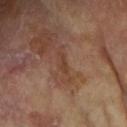Part of a total-body skin-imaging series; this lesion was reviewed on a skin check and was not flagged for biopsy. Cropped from a whole-body photographic skin survey; the tile spans about 15 mm. Imaged with cross-polarized lighting. Automated image analysis of the tile measured roughly 6 lightness units darker than nearby skin and a normalized border contrast of about 5.5. The software also gave border irregularity of about 4.5 on a 0–10 scale. And it measured a classifier nevus-likeness of about 0/100 and lesion-presence confidence of about 75/100. The lesion is on the arm. Approximately 2.5 mm at its widest. A female patient, approximately 75 years of age.